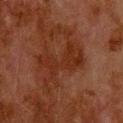Clinical impression: Imaged during a routine full-body skin examination; the lesion was not biopsied and no histopathology is available. Context: Located on the back. Captured under cross-polarized illumination. The recorded lesion diameter is about 6 mm. The patient is a male aged 78 to 82. A 15 mm crop from a total-body photograph taken for skin-cancer surveillance. Automated image analysis of the tile measured an average lesion color of about L≈23 a*≈21 b*≈25 (CIELAB), about 5 CIELAB-L* units darker than the surrounding skin, and a lesion-to-skin contrast of about 6 (normalized; higher = more distinct). It also reported a border-irregularity rating of about 7/10 and a color-variation rating of about 2.5/10.